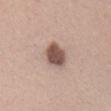biopsy_status: not biopsied; imaged during a skin examination
patient:
  sex: female
  age_approx: 25
site: head or neck
lesion_size:
  long_diameter_mm_approx: 3.5
automated_metrics:
  shape_asymmetry: 0.2
image:
  source: total-body photography crop
  field_of_view_mm: 15